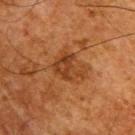workup = imaged on a skin check; not biopsied | body site = the upper back | illumination = cross-polarized illumination | image source = ~15 mm tile from a whole-body skin photo | diameter = ~3.5 mm (longest diameter) | subject = male, aged around 65.